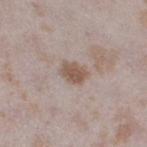{"biopsy_status": "not biopsied; imaged during a skin examination", "image": {"source": "total-body photography crop", "field_of_view_mm": 15}, "site": "right thigh", "patient": {"sex": "female", "age_approx": 25}}